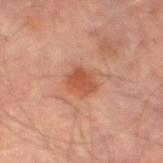Q: Was a biopsy performed?
A: catalogued during a skin exam; not biopsied
Q: What are the patient's age and sex?
A: male, aged 63 to 67
Q: Where on the body is the lesion?
A: the left thigh
Q: How was this image acquired?
A: 15 mm crop, total-body photography
Q: How large is the lesion?
A: ≈3 mm